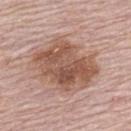body site = the upper back
lesion size = about 8 mm
illumination = white-light
subject = female, approximately 65 years of age
acquisition = total-body-photography crop, ~15 mm field of view